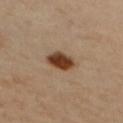The lesion was tiled from a total-body skin photograph and was not biopsied. A 15 mm close-up extracted from a 3D total-body photography capture. The patient is a female roughly 45 years of age. This is a cross-polarized tile. Longest diameter approximately 3.5 mm. From the right upper arm.